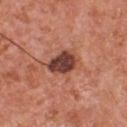The lesion was tiled from a total-body skin photograph and was not biopsied.
The lesion's longest dimension is about 3.5 mm.
The lesion is located on the chest.
A close-up tile cropped from a whole-body skin photograph, about 15 mm across.
This is a white-light tile.
The total-body-photography lesion software estimated a mean CIELAB color near L≈41 a*≈25 b*≈26, roughly 17 lightness units darker than nearby skin, and a normalized lesion–skin contrast near 12.5. The analysis additionally found a lesion-detection confidence of about 100/100.
The subject is a male approximately 55 years of age.